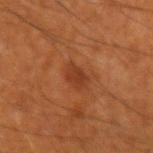biopsy status = total-body-photography surveillance lesion; no biopsy
image-analysis metrics = a mean CIELAB color near L≈36 a*≈26 b*≈33, a lesion–skin lightness drop of about 7, and a normalized lesion–skin contrast near 6.5; border irregularity of about 2 on a 0–10 scale and peripheral color asymmetry of about 0.5; an automated nevus-likeness rating near 10 out of 100 and lesion-presence confidence of about 100/100
body site = the arm
subject = male, approximately 60 years of age
image source = ~15 mm crop, total-body skin-cancer survey